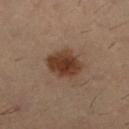Impression: Part of a total-body skin-imaging series; this lesion was reviewed on a skin check and was not flagged for biopsy. Acquisition and patient details: Captured under cross-polarized illumination. The patient is a male aged approximately 30. This image is a 15 mm lesion crop taken from a total-body photograph. The lesion-visualizer software estimated a lesion color around L≈29 a*≈15 b*≈23 in CIELAB, about 10 CIELAB-L* units darker than the surrounding skin, and a normalized lesion–skin contrast near 11. The analysis additionally found a border-irregularity index near 1.5/10 and internal color variation of about 3 on a 0–10 scale. The lesion is on the left lower leg. Approximately 3.5 mm at its widest.